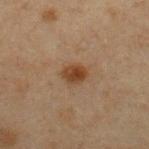<tbp_lesion>
  <biopsy_status>not biopsied; imaged during a skin examination</biopsy_status>
  <lesion_size>
    <long_diameter_mm_approx>2.5</long_diameter_mm_approx>
  </lesion_size>
  <site>chest</site>
  <lighting>cross-polarized</lighting>
  <image>
    <source>total-body photography crop</source>
    <field_of_view_mm>15</field_of_view_mm>
  </image>
  <automated_metrics>
    <area_mm2_approx>4.5</area_mm2_approx>
    <eccentricity>0.6</eccentricity>
    <shape_asymmetry>0.15</shape_asymmetry>
  </automated_metrics>
  <patient>
    <sex>male</sex>
    <age_approx>60</age_approx>
  </patient>
</tbp_lesion>